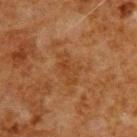biopsy status = catalogued during a skin exam; not biopsied | diameter = ~4 mm (longest diameter) | illumination = cross-polarized illumination | image = total-body-photography crop, ~15 mm field of view | subject = male, aged around 80 | image-analysis metrics = a classifier nevus-likeness of about 0/100 and a lesion-detection confidence of about 100/100 | site = the upper back.